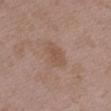<record>
  <biopsy_status>not biopsied; imaged during a skin examination</biopsy_status>
  <site>chest</site>
  <automated_metrics>
    <area_mm2_approx>7.0</area_mm2_approx>
    <eccentricity>0.6</eccentricity>
    <shape_asymmetry>0.25</shape_asymmetry>
    <border_irregularity_0_10>2.5</border_irregularity_0_10>
    <color_variation_0_10>2.0</color_variation_0_10>
    <peripheral_color_asymmetry>0.5</peripheral_color_asymmetry>
  </automated_metrics>
  <patient>
    <sex>female</sex>
    <age_approx>35</age_approx>
  </patient>
  <image>
    <source>total-body photography crop</source>
    <field_of_view_mm>15</field_of_view_mm>
  </image>
</record>